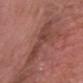* follow-up — imaged on a skin check; not biopsied
* patient — male, roughly 65 years of age
* anatomic site — the head or neck
* lighting — white-light
* image-analysis metrics — border irregularity of about 6 on a 0–10 scale, internal color variation of about 3 on a 0–10 scale, and peripheral color asymmetry of about 1
* image — ~15 mm tile from a whole-body skin photo
* lesion size — ~5.5 mm (longest diameter)